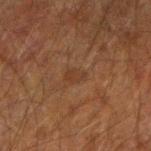anatomic site: the right forearm | diameter: ≈2.5 mm | automated lesion analysis: a detector confidence of about 100 out of 100 that the crop contains a lesion | imaging modality: ~15 mm tile from a whole-body skin photo | illumination: cross-polarized | patient: male, aged around 50.